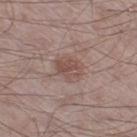– biopsy status · imaged on a skin check; not biopsied
– site · the left thigh
– subject · male, in their mid-60s
– illumination · white-light
– size · ~3.5 mm (longest diameter)
– image · ~15 mm tile from a whole-body skin photo
– TBP lesion metrics · a footprint of about 8.5 mm² and a symmetry-axis asymmetry near 0.2; an average lesion color of about L≈50 a*≈18 b*≈22 (CIELAB) and a normalized lesion–skin contrast near 6; border irregularity of about 2 on a 0–10 scale, a color-variation rating of about 3.5/10, and peripheral color asymmetry of about 1; a nevus-likeness score of about 5/100 and a lesion-detection confidence of about 100/100